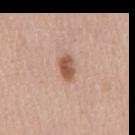follow-up: imaged on a skin check; not biopsied
patient: female, aged approximately 45
imaging modality: ~15 mm tile from a whole-body skin photo
anatomic site: the mid back
TBP lesion metrics: a footprint of about 5 mm², an outline eccentricity of about 0.85 (0 = round, 1 = elongated), and a symmetry-axis asymmetry near 0.25; border irregularity of about 2.5 on a 0–10 scale and radial color variation of about 1